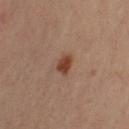Clinical impression:
This lesion was catalogued during total-body skin photography and was not selected for biopsy.
Acquisition and patient details:
A female patient, about 35 years old. Located on the left upper arm. Longest diameter approximately 2.5 mm. A roughly 15 mm field-of-view crop from a total-body skin photograph.